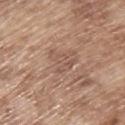<lesion>
  <biopsy_status>not biopsied; imaged during a skin examination</biopsy_status>
  <lighting>white-light</lighting>
  <image>
    <source>total-body photography crop</source>
    <field_of_view_mm>15</field_of_view_mm>
  </image>
  <site>upper back</site>
  <patient>
    <sex>male</sex>
    <age_approx>65</age_approx>
  </patient>
  <lesion_size>
    <long_diameter_mm_approx>5.0</long_diameter_mm_approx>
  </lesion_size>
</lesion>